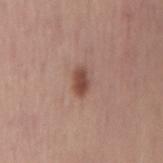Case summary:
– workup · no biopsy performed (imaged during a skin exam)
– TBP lesion metrics · a within-lesion color-variation index near 2.5/10 and a peripheral color-asymmetry measure near 1; a classifier nevus-likeness of about 95/100
– patient · female, about 55 years old
– image source · ~15 mm crop, total-body skin-cancer survey
– site · the mid back
– lesion diameter · ~3 mm (longest diameter)